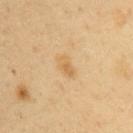Clinical impression:
Imaged during a routine full-body skin examination; the lesion was not biopsied and no histopathology is available.
Acquisition and patient details:
A 15 mm crop from a total-body photograph taken for skin-cancer surveillance. The lesion is located on the abdomen. Automated image analysis of the tile measured a shape eccentricity near 0.8 and a symmetry-axis asymmetry near 0.2. And it measured a border-irregularity index near 2/10 and peripheral color asymmetry of about 1. It also reported a classifier nevus-likeness of about 5/100 and a lesion-detection confidence of about 100/100. A male subject aged 48 to 52. Approximately 3 mm at its widest. The tile uses cross-polarized illumination.